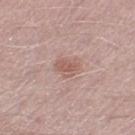  biopsy_status: not biopsied; imaged during a skin examination
  image:
    source: total-body photography crop
    field_of_view_mm: 15
  site: right lower leg
  automated_metrics:
    area_mm2_approx: 5.0
    eccentricity: 0.65
    vs_skin_darker_L: 8.0
    vs_skin_contrast_norm: 6.0
  lesion_size:
    long_diameter_mm_approx: 3.0
  lighting: white-light
  patient:
    sex: male
    age_approx: 50